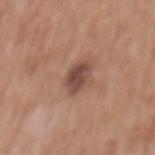follow-up=catalogued during a skin exam; not biopsied.